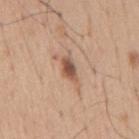The lesion was photographed on a routine skin check and not biopsied; there is no pathology result.
A male patient, aged around 65.
About 3.5 mm across.
On the mid back.
A roughly 15 mm field-of-view crop from a total-body skin photograph.
The total-body-photography lesion software estimated about 14 CIELAB-L* units darker than the surrounding skin and a normalized border contrast of about 9. The software also gave a border-irregularity index near 3/10 and internal color variation of about 4 on a 0–10 scale.